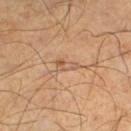anatomic site: the left lower leg | subject: male, roughly 55 years of age | image source: 15 mm crop, total-body photography.